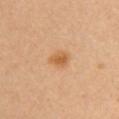workup: no biopsy performed (imaged during a skin exam); patient: female, aged around 25; size: ≈2.5 mm; site: the chest; image: total-body-photography crop, ~15 mm field of view; illumination: cross-polarized illumination.